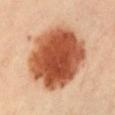The lesion was photographed on a routine skin check and not biopsied; there is no pathology result.
A lesion tile, about 15 mm wide, cut from a 3D total-body photograph.
The patient is a female approximately 60 years of age.
From the abdomen.
Imaged with cross-polarized lighting.
Approximately 8 mm at its widest.